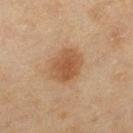The lesion was tiled from a total-body skin photograph and was not biopsied. On the left thigh. This image is a 15 mm lesion crop taken from a total-body photograph. This is a cross-polarized tile. The patient is a female roughly 60 years of age. About 4 mm across.